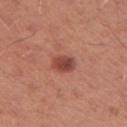{
  "biopsy_status": "not biopsied; imaged during a skin examination",
  "image": {
    "source": "total-body photography crop",
    "field_of_view_mm": 15
  },
  "patient": {
    "sex": "male",
    "age_approx": 30
  },
  "lesion_size": {
    "long_diameter_mm_approx": 3.0
  },
  "lighting": "white-light",
  "site": "left upper arm"
}